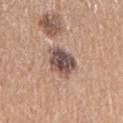workup — total-body-photography surveillance lesion; no biopsy | body site — the right upper arm | subject — female, in their mid- to late 60s | image-analysis metrics — a shape eccentricity near 0.5; a lesion color around L≈50 a*≈17 b*≈21 in CIELAB | imaging modality — 15 mm crop, total-body photography | size — ≈4 mm.